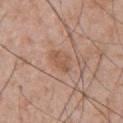Image and clinical context:
The subject is a male aged around 55. Imaged with white-light lighting. The lesion is located on the upper back. A close-up tile cropped from a whole-body skin photograph, about 15 mm across.